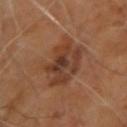No biopsy was performed on this lesion — it was imaged during a full skin examination and was not determined to be concerning. This is a cross-polarized tile. The recorded lesion diameter is about 5.5 mm. A male subject aged 68 to 72. A roughly 15 mm field-of-view crop from a total-body skin photograph. The lesion-visualizer software estimated a footprint of about 18 mm², a shape eccentricity near 0.65, and a shape-asymmetry score of about 0.25 (0 = symmetric). And it measured a detector confidence of about 100 out of 100 that the crop contains a lesion. From the right upper arm.